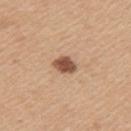Findings:
- imaging modality · total-body-photography crop, ~15 mm field of view
- illumination · white-light illumination
- location · the left upper arm
- subject · female, in their 40s